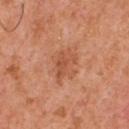  biopsy_status: not biopsied; imaged during a skin examination
  site: front of the torso
  patient:
    sex: male
    age_approx: 55
  lighting: white-light
  image:
    source: total-body photography crop
    field_of_view_mm: 15
  lesion_size:
    long_diameter_mm_approx: 4.0